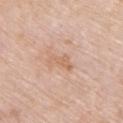The tile uses white-light illumination.
The lesion is on the upper back.
The recorded lesion diameter is about 3 mm.
A female patient, aged 68 to 72.
The total-body-photography lesion software estimated a footprint of about 4 mm².
A close-up tile cropped from a whole-body skin photograph, about 15 mm across.